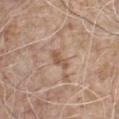Q: Was a biopsy performed?
A: imaged on a skin check; not biopsied
Q: What is the imaging modality?
A: ~15 mm crop, total-body skin-cancer survey
Q: Lesion location?
A: the chest
Q: How large is the lesion?
A: ≈2.5 mm
Q: What did automated image analysis measure?
A: border irregularity of about 4 on a 0–10 scale, internal color variation of about 1 on a 0–10 scale, and radial color variation of about 0.5; a nevus-likeness score of about 0/100 and a detector confidence of about 100 out of 100 that the crop contains a lesion
Q: Patient demographics?
A: male, in their mid-50s
Q: Illumination type?
A: white-light